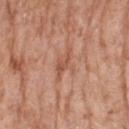Assessment: The lesion was tiled from a total-body skin photograph and was not biopsied. Clinical summary: A roughly 15 mm field-of-view crop from a total-body skin photograph. The recorded lesion diameter is about 4 mm. Automated tile analysis of the lesion measured a mean CIELAB color near L≈55 a*≈24 b*≈32, about 8 CIELAB-L* units darker than the surrounding skin, and a normalized border contrast of about 5.5. It also reported a classifier nevus-likeness of about 0/100. This is a white-light tile. From the right forearm. The patient is a female about 75 years old.